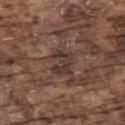follow-up: imaged on a skin check; not biopsied | image: 15 mm crop, total-body photography | location: the upper back | size: ≈3 mm | subject: male, aged 73–77 | tile lighting: white-light | automated metrics: a lesion–skin lightness drop of about 8; a nevus-likeness score of about 0/100 and lesion-presence confidence of about 70/100.